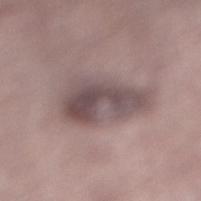Notes:
- notes — catalogued during a skin exam; not biopsied
- site — the leg
- tile lighting — white-light
- patient — male, aged 53 to 57
- image source — total-body-photography crop, ~15 mm field of view